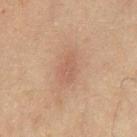Q: Was a biopsy performed?
A: total-body-photography surveillance lesion; no biopsy
Q: What are the patient's age and sex?
A: male, aged around 60
Q: Automated lesion metrics?
A: an area of roughly 6 mm², an eccentricity of roughly 0.8, and a symmetry-axis asymmetry near 0.35; a border-irregularity rating of about 3/10 and a within-lesion color-variation index near 2.5/10; a nevus-likeness score of about 10/100 and lesion-presence confidence of about 100/100
Q: What is the lesion's diameter?
A: about 3.5 mm
Q: How was this image acquired?
A: ~15 mm crop, total-body skin-cancer survey